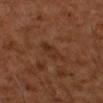| key | value |
|---|---|
| follow-up | catalogued during a skin exam; not biopsied |
| imaging modality | 15 mm crop, total-body photography |
| patient | male, in their 60s |
| location | the right upper arm |
| tile lighting | cross-polarized |
| automated lesion analysis | a nevus-likeness score of about 0/100 and a detector confidence of about 100 out of 100 that the crop contains a lesion |